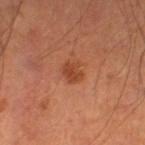Assessment: Recorded during total-body skin imaging; not selected for excision or biopsy. Acquisition and patient details: The patient is a male aged 53–57. Imaged with cross-polarized lighting. Located on the right lower leg. Cropped from a whole-body photographic skin survey; the tile spans about 15 mm.